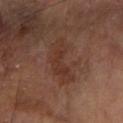Case summary:
– follow-up · catalogued during a skin exam; not biopsied
– imaging modality · ~15 mm tile from a whole-body skin photo
– patient · male, aged approximately 85 or older
– anatomic site · the arm
– size · ~5.5 mm (longest diameter)
– illumination · cross-polarized illumination